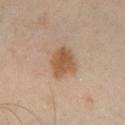The lesion was tiled from a total-body skin photograph and was not biopsied.